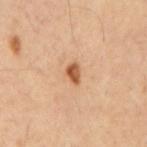Case summary:
* workup: imaged on a skin check; not biopsied
* lesion size: ≈2.5 mm
* tile lighting: cross-polarized
* body site: the mid back
* image: ~15 mm crop, total-body skin-cancer survey
* subject: male, aged around 60
* TBP lesion metrics: a footprint of about 3 mm²; an average lesion color of about L≈53 a*≈24 b*≈37 (CIELAB) and a normalized border contrast of about 10; border irregularity of about 2.5 on a 0–10 scale, a within-lesion color-variation index near 3.5/10, and radial color variation of about 1.5